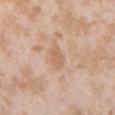The lesion was photographed on a routine skin check and not biopsied; there is no pathology result.